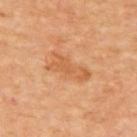The lesion was photographed on a routine skin check and not biopsied; there is no pathology result. This is a cross-polarized tile. A close-up tile cropped from a whole-body skin photograph, about 15 mm across. On the upper back. A patient about 65 years old. Measured at roughly 5 mm in maximum diameter.